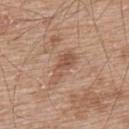Assessment:
Imaged during a routine full-body skin examination; the lesion was not biopsied and no histopathology is available.
Background:
The lesion is on the upper back. Imaged with white-light lighting. A male patient, aged approximately 65. A 15 mm close-up extracted from a 3D total-body photography capture. Approximately 3.5 mm at its widest.